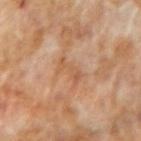follow-up — no biopsy performed (imaged during a skin exam)
size — ~3 mm (longest diameter)
acquisition — total-body-photography crop, ~15 mm field of view
illumination — cross-polarized illumination
subject — male, approximately 70 years of age
automated metrics — an outline eccentricity of about 0.9 (0 = round, 1 = elongated) and a symmetry-axis asymmetry near 0.5; an average lesion color of about L≈55 a*≈21 b*≈34 (CIELAB), roughly 7 lightness units darker than nearby skin, and a normalized lesion–skin contrast near 5.5; a classifier nevus-likeness of about 0/100
body site — the left forearm